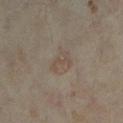Recorded during total-body skin imaging; not selected for excision or biopsy. Cropped from a whole-body photographic skin survey; the tile spans about 15 mm. The subject is a female aged approximately 35. Located on the left lower leg.